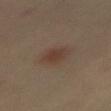Notes:
– workup: total-body-photography surveillance lesion; no biopsy
– patient: female, about 65 years old
– lesion diameter: ≈3.5 mm
– imaging modality: total-body-photography crop, ~15 mm field of view
– tile lighting: cross-polarized illumination
– automated metrics: an eccentricity of roughly 0.75 and a shape-asymmetry score of about 0.25 (0 = symmetric); border irregularity of about 2.5 on a 0–10 scale, a color-variation rating of about 2.5/10, and radial color variation of about 1
– location: the abdomen